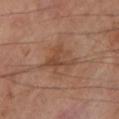Case summary:
• follow-up · total-body-photography surveillance lesion; no biopsy
• patient · male, in their 70s
• lighting · cross-polarized
• lesion size · ≈5 mm
• image source · 15 mm crop, total-body photography
• automated lesion analysis · an average lesion color of about L≈44 a*≈19 b*≈29 (CIELAB), roughly 7 lightness units darker than nearby skin, and a lesion-to-skin contrast of about 6 (normalized; higher = more distinct); a border-irregularity rating of about 6.5/10; a nevus-likeness score of about 5/100 and a lesion-detection confidence of about 100/100
• body site · the left lower leg